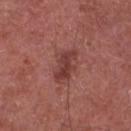notes: total-body-photography surveillance lesion; no biopsy | patient: male, about 65 years old | lesion size: about 4 mm | site: the chest | automated lesion analysis: a footprint of about 7 mm², an eccentricity of roughly 0.9, and a shape-asymmetry score of about 0.3 (0 = symmetric); a peripheral color-asymmetry measure near 1 | acquisition: 15 mm crop, total-body photography | lighting: white-light.